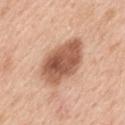workup: total-body-photography surveillance lesion; no biopsy | patient: male, about 50 years old | site: the back | lesion size: ~6.5 mm (longest diameter) | illumination: white-light illumination | TBP lesion metrics: an area of roughly 21 mm², an outline eccentricity of about 0.8 (0 = round, 1 = elongated), and two-axis asymmetry of about 0.15; an automated nevus-likeness rating near 35 out of 100 and a detector confidence of about 100 out of 100 that the crop contains a lesion | imaging modality: total-body-photography crop, ~15 mm field of view.